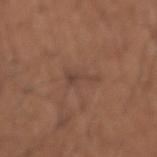From the left forearm.
An algorithmic analysis of the crop reported an average lesion color of about L≈42 a*≈18 b*≈25 (CIELAB), about 6 CIELAB-L* units darker than the surrounding skin, and a normalized lesion–skin contrast near 5.5. The analysis additionally found an automated nevus-likeness rating near 0 out of 100 and a lesion-detection confidence of about 100/100.
The patient is a male approximately 65 years of age.
A 15 mm close-up extracted from a 3D total-body photography capture.
Longest diameter approximately 3.5 mm.